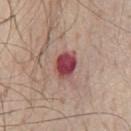Q: Automated lesion metrics?
A: a footprint of about 7.5 mm², an outline eccentricity of about 0.65 (0 = round, 1 = elongated), and a shape-asymmetry score of about 0.2 (0 = symmetric); an average lesion color of about L≈45 a*≈30 b*≈21 (CIELAB), a lesion–skin lightness drop of about 16, and a lesion-to-skin contrast of about 11.5 (normalized; higher = more distinct)
Q: What is the anatomic site?
A: the front of the torso
Q: What are the patient's age and sex?
A: male, in their 80s
Q: How was this image acquired?
A: ~15 mm tile from a whole-body skin photo
Q: Lesion size?
A: ~3.5 mm (longest diameter)